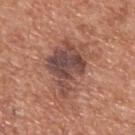This lesion was catalogued during total-body skin photography and was not selected for biopsy.
Measured at roughly 8 mm in maximum diameter.
Cropped from a total-body skin-imaging series; the visible field is about 15 mm.
The total-body-photography lesion software estimated a border-irregularity rating of about 5.5/10, a within-lesion color-variation index near 6.5/10, and radial color variation of about 2. The software also gave a classifier nevus-likeness of about 45/100 and a lesion-detection confidence of about 100/100.
Captured under white-light illumination.
The lesion is on the upper back.
A male patient aged 63 to 67.